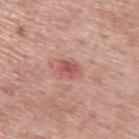No biopsy was performed on this lesion — it was imaged during a full skin examination and was not determined to be concerning. From the back. The tile uses white-light illumination. A male subject, aged 73–77. About 2.5 mm across. A close-up tile cropped from a whole-body skin photograph, about 15 mm across.